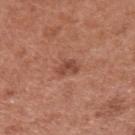Clinical impression:
Part of a total-body skin-imaging series; this lesion was reviewed on a skin check and was not flagged for biopsy.
Context:
A lesion tile, about 15 mm wide, cut from a 3D total-body photograph. A male subject, in their mid- to late 50s. On the upper back.